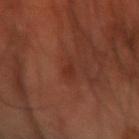biopsy_status: not biopsied; imaged during a skin examination
patient:
  sex: male
  age_approx: 70
lighting: cross-polarized
site: right forearm
image:
  source: total-body photography crop
  field_of_view_mm: 15